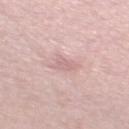follow-up=imaged on a skin check; not biopsied
site=the left thigh
image-analysis metrics=an average lesion color of about L≈67 a*≈20 b*≈20 (CIELAB), about 8 CIELAB-L* units darker than the surrounding skin, and a normalized lesion–skin contrast near 4.5
imaging modality=15 mm crop, total-body photography
subject=male, aged 58 to 62
illumination=white-light illumination